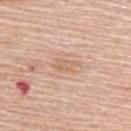workup: catalogued during a skin exam; not biopsied
patient: female, aged 63 to 67
imaging modality: ~15 mm crop, total-body skin-cancer survey
diameter: about 3 mm
location: the upper back
tile lighting: white-light